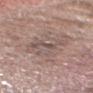notes: catalogued during a skin exam; not biopsied | tile lighting: white-light illumination | TBP lesion metrics: a border-irregularity index near 8.5/10, a within-lesion color-variation index near 4/10, and radial color variation of about 1.5 | patient: male, aged 68 to 72 | image source: ~15 mm tile from a whole-body skin photo | anatomic site: the head or neck.